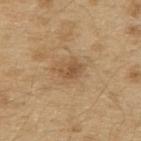Assessment:
This lesion was catalogued during total-body skin photography and was not selected for biopsy.
Background:
A male subject aged 68 to 72. The lesion is on the upper back. A 15 mm close-up tile from a total-body photography series done for melanoma screening. Measured at roughly 2.5 mm in maximum diameter. Imaged with white-light lighting.